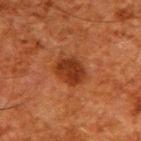Impression: The lesion was tiled from a total-body skin photograph and was not biopsied. Image and clinical context: The patient is a male approximately 60 years of age. A roughly 15 mm field-of-view crop from a total-body skin photograph. From the upper back.